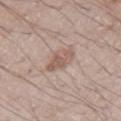{
  "biopsy_status": "not biopsied; imaged during a skin examination",
  "site": "left upper arm",
  "patient": {
    "sex": "male",
    "age_approx": 70
  },
  "lighting": "white-light",
  "lesion_size": {
    "long_diameter_mm_approx": 3.0
  },
  "image": {
    "source": "total-body photography crop",
    "field_of_view_mm": 15
  },
  "automated_metrics": {
    "area_mm2_approx": 5.5,
    "shape_asymmetry": 0.3,
    "cielab_L": 56,
    "cielab_a": 17,
    "cielab_b": 25,
    "vs_skin_darker_L": 9.0,
    "vs_skin_contrast_norm": 6.5,
    "nevus_likeness_0_100": 65,
    "lesion_detection_confidence_0_100": 100
  }
}